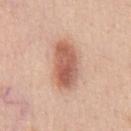notes — total-body-photography surveillance lesion; no biopsy
location — the chest
image source — ~15 mm tile from a whole-body skin photo
diameter — ≈5.5 mm
illumination — white-light
subject — male, aged around 50